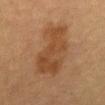  biopsy_status: not biopsied; imaged during a skin examination
  lesion_size:
    long_diameter_mm_approx: 7.0
  site: abdomen
  lighting: cross-polarized
  image:
    source: total-body photography crop
    field_of_view_mm: 15
  patient:
    sex: female
    age_approx: 40